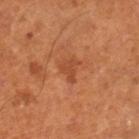No biopsy was performed on this lesion — it was imaged during a full skin examination and was not determined to be concerning.
The total-body-photography lesion software estimated an area of roughly 3.5 mm², an eccentricity of roughly 0.7, and a symmetry-axis asymmetry near 0.6. The analysis additionally found a mean CIELAB color near L≈46 a*≈28 b*≈37, about 7 CIELAB-L* units darker than the surrounding skin, and a normalized lesion–skin contrast near 5.5. It also reported an automated nevus-likeness rating near 0 out of 100 and a detector confidence of about 100 out of 100 that the crop contains a lesion.
The tile uses cross-polarized illumination.
This image is a 15 mm lesion crop taken from a total-body photograph.
The patient is a male aged 63–67.
The lesion is located on the left leg.
Approximately 2.5 mm at its widest.